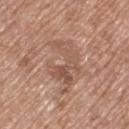  biopsy_status: not biopsied; imaged during a skin examination
  lighting: white-light
  patient:
    sex: female
    age_approx: 75
  site: left thigh
  lesion_size:
    long_diameter_mm_approx: 6.5
  image:
    source: total-body photography crop
    field_of_view_mm: 15